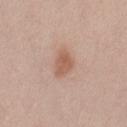Cropped from a whole-body photographic skin survey; the tile spans about 15 mm. Captured under white-light illumination. From the left thigh. Measured at roughly 3 mm in maximum diameter. The subject is a female roughly 40 years of age.